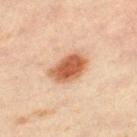Notes:
– notes — catalogued during a skin exam; not biopsied
– lesion diameter — ~4 mm (longest diameter)
– image — total-body-photography crop, ~15 mm field of view
– subject — male, aged 58 to 62
– illumination — cross-polarized
– anatomic site — the left thigh